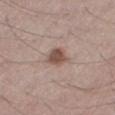A male patient, in their 50s. A close-up tile cropped from a whole-body skin photograph, about 15 mm across. The lesion is on the right thigh. About 3 mm across. The total-body-photography lesion software estimated an automated nevus-likeness rating near 90 out of 100 and a lesion-detection confidence of about 100/100. This is a white-light tile.